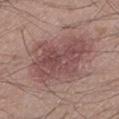• biopsy status · total-body-photography surveillance lesion; no biopsy
• imaging modality · ~15 mm crop, total-body skin-cancer survey
• subject · male, aged 18 to 22
• site · the left lower leg